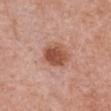Imaged during a routine full-body skin examination; the lesion was not biopsied and no histopathology is available.
A 15 mm close-up tile from a total-body photography series done for melanoma screening.
A female subject, about 50 years old.
The lesion is on the chest.